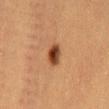Imaged during a routine full-body skin examination; the lesion was not biopsied and no histopathology is available.
The tile uses cross-polarized illumination.
A female subject, about 40 years old.
A 15 mm close-up extracted from a 3D total-body photography capture.
Longest diameter approximately 3 mm.
The total-body-photography lesion software estimated a lesion color around L≈36 a*≈22 b*≈31 in CIELAB, about 14 CIELAB-L* units darker than the surrounding skin, and a normalized lesion–skin contrast near 11.5. The analysis additionally found border irregularity of about 1.5 on a 0–10 scale, a color-variation rating of about 4.5/10, and radial color variation of about 1.5. The software also gave a nevus-likeness score of about 100/100 and a lesion-detection confidence of about 100/100.
The lesion is located on the abdomen.